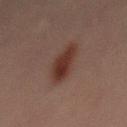{"biopsy_status": "not biopsied; imaged during a skin examination", "patient": {"sex": "male", "age_approx": 30}, "image": {"source": "total-body photography crop", "field_of_view_mm": 15}, "site": "mid back"}